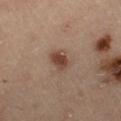Assessment: The lesion was tiled from a total-body skin photograph and was not biopsied. Background: Longest diameter approximately 3 mm. A region of skin cropped from a whole-body photographic capture, roughly 15 mm wide. Automated image analysis of the tile measured an area of roughly 5 mm² and an outline eccentricity of about 0.8 (0 = round, 1 = elongated). The analysis additionally found a lesion color around L≈42 a*≈19 b*≈26 in CIELAB and about 11 CIELAB-L* units darker than the surrounding skin. It also reported a color-variation rating of about 3/10. This is a cross-polarized tile. The subject is a male aged 53 to 57. From the right thigh.